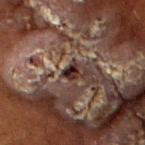Findings:
* workup — imaged on a skin check; not biopsied
* size — ~2 mm (longest diameter)
* image source — total-body-photography crop, ~15 mm field of view
* subject — female, aged 78–82
* body site — the left upper arm
* image-analysis metrics — a lesion area of about 3 mm² and a shape eccentricity near 0.65; a lesion–skin lightness drop of about 12; border irregularity of about 2.5 on a 0–10 scale, internal color variation of about 5.5 on a 0–10 scale, and radial color variation of about 2